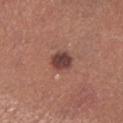workup: catalogued during a skin exam; not biopsied | lesion diameter: about 3 mm | body site: the left lower leg | image: 15 mm crop, total-body photography | patient: female, aged 63 to 67.